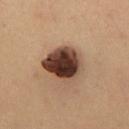biopsy status: no biopsy performed (imaged during a skin exam)
diameter: ≈4.5 mm
image-analysis metrics: an average lesion color of about L≈39 a*≈19 b*≈26 (CIELAB) and a lesion–skin lightness drop of about 22; a peripheral color-asymmetry measure near 2.5; a nevus-likeness score of about 85/100 and lesion-presence confidence of about 100/100
image source: 15 mm crop, total-body photography
illumination: cross-polarized illumination
site: the chest
patient: male, aged around 50